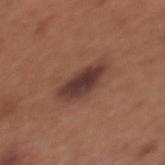Q: Was a biopsy performed?
A: imaged on a skin check; not biopsied